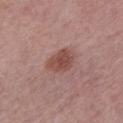notes=catalogued during a skin exam; not biopsied.